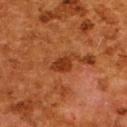No biopsy was performed on this lesion — it was imaged during a full skin examination and was not determined to be concerning. From the upper back. A female subject, aged 48 to 52. This image is a 15 mm lesion crop taken from a total-body photograph.